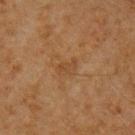lesion size: about 2.5 mm
automated metrics: a border-irregularity rating of about 4/10, internal color variation of about 1.5 on a 0–10 scale, and a peripheral color-asymmetry measure near 0.5; an automated nevus-likeness rating near 0 out of 100
location: the arm
subject: male, roughly 65 years of age
imaging modality: total-body-photography crop, ~15 mm field of view
illumination: cross-polarized